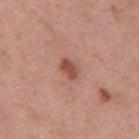Assessment: No biopsy was performed on this lesion — it was imaged during a full skin examination and was not determined to be concerning. Clinical summary: From the mid back. A 15 mm close-up extracted from a 3D total-body photography capture. The lesion-visualizer software estimated an average lesion color of about L≈51 a*≈26 b*≈28 (CIELAB) and roughly 11 lightness units darker than nearby skin. The analysis additionally found border irregularity of about 2 on a 0–10 scale, a within-lesion color-variation index near 3/10, and peripheral color asymmetry of about 1. The patient is a male aged 38–42. Measured at roughly 2.5 mm in maximum diameter.